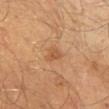biopsy status = catalogued during a skin exam; not biopsied | size = ≈3 mm | subject = male, aged approximately 40 | location = the right lower leg | automated lesion analysis = a nevus-likeness score of about 10/100 and lesion-presence confidence of about 100/100 | imaging modality = 15 mm crop, total-body photography.